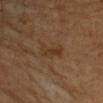Clinical impression:
Imaged during a routine full-body skin examination; the lesion was not biopsied and no histopathology is available.
Background:
Longest diameter approximately 4 mm. A 15 mm crop from a total-body photograph taken for skin-cancer surveillance. The patient is a male aged approximately 70. Located on the arm.